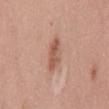Q: What did automated image analysis measure?
A: an area of roughly 5.5 mm², an eccentricity of roughly 0.95, and a symmetry-axis asymmetry near 0.25; a border-irregularity rating of about 3.5/10, internal color variation of about 3.5 on a 0–10 scale, and peripheral color asymmetry of about 1; a classifier nevus-likeness of about 60/100 and lesion-presence confidence of about 100/100
Q: What kind of image is this?
A: ~15 mm crop, total-body skin-cancer survey
Q: Patient demographics?
A: female, aged 33 to 37
Q: Illumination type?
A: white-light
Q: Lesion location?
A: the mid back
Q: What is the lesion's diameter?
A: ≈4.5 mm